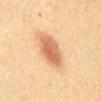On the abdomen.
The recorded lesion diameter is about 4.5 mm.
This is a cross-polarized tile.
A 15 mm close-up tile from a total-body photography series done for melanoma screening.
An algorithmic analysis of the crop reported an area of roughly 12 mm² and a symmetry-axis asymmetry near 0.15.
A female patient, about 50 years old.